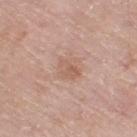lighting: white-light
automated_metrics:
  area_mm2_approx: 6.0
  eccentricity: 0.6
  shape_asymmetry: 0.2
  vs_skin_darker_L: 7.0
  vs_skin_contrast_norm: 5.0
  border_irregularity_0_10: 2.0
  color_variation_0_10: 3.5
  peripheral_color_asymmetry: 1.5
  nevus_likeness_0_100: 0
image:
  source: total-body photography crop
  field_of_view_mm: 15
site: right thigh
patient:
  sex: male
  age_approx: 80